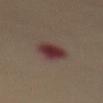Clinical impression:
Imaged during a routine full-body skin examination; the lesion was not biopsied and no histopathology is available.
Acquisition and patient details:
A female subject, aged approximately 70. Located on the abdomen. This image is a 15 mm lesion crop taken from a total-body photograph.